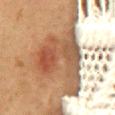This lesion was catalogued during total-body skin photography and was not selected for biopsy. A close-up tile cropped from a whole-body skin photograph, about 15 mm across. The subject is a female approximately 50 years of age. On the lower back. Imaged with cross-polarized lighting.